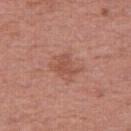No biopsy was performed on this lesion — it was imaged during a full skin examination and was not determined to be concerning.